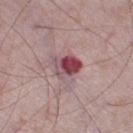{"biopsy_status": "not biopsied; imaged during a skin examination", "image": {"source": "total-body photography crop", "field_of_view_mm": 15}, "site": "left thigh", "lighting": "white-light", "patient": {"sex": "male", "age_approx": 75}, "lesion_size": {"long_diameter_mm_approx": 3.5}}